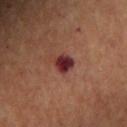No biopsy was performed on this lesion — it was imaged during a full skin examination and was not determined to be concerning.
Located on the arm.
Captured under cross-polarized illumination.
The patient is a male about 65 years old.
A roughly 15 mm field-of-view crop from a total-body skin photograph.
The lesion-visualizer software estimated an area of roughly 5.5 mm², an outline eccentricity of about 0.7 (0 = round, 1 = elongated), and a shape-asymmetry score of about 0.3 (0 = symmetric). The analysis additionally found about 13 CIELAB-L* units darker than the surrounding skin and a normalized border contrast of about 13. The analysis additionally found a border-irregularity rating of about 3.5/10 and radial color variation of about 1.5.